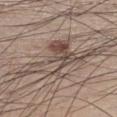subject = male, in their mid-50s
tile lighting = white-light illumination
diameter = about 9.5 mm
imaging modality = total-body-photography crop, ~15 mm field of view
automated lesion analysis = an eccentricity of roughly 0.9; a lesion–skin lightness drop of about 10 and a normalized border contrast of about 7.5; a nevus-likeness score of about 5/100 and lesion-presence confidence of about 80/100
location = the leg